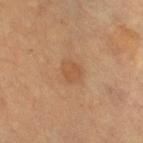Recorded during total-body skin imaging; not selected for excision or biopsy. Cropped from a total-body skin-imaging series; the visible field is about 15 mm. On the right lower leg. A female subject, aged 68 to 72. Longest diameter approximately 2.5 mm. Automated tile analysis of the lesion measured two-axis asymmetry of about 0.15. It also reported a lesion color around L≈51 a*≈20 b*≈34 in CIELAB and a normalized border contrast of about 5.5. The analysis additionally found a nevus-likeness score of about 5/100.